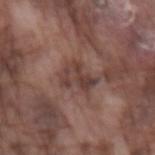follow-up=catalogued during a skin exam; not biopsied | automated lesion analysis=a classifier nevus-likeness of about 0/100 and a detector confidence of about 95 out of 100 that the crop contains a lesion | imaging modality=15 mm crop, total-body photography | lesion diameter=~4 mm (longest diameter) | patient=male, in their mid- to late 70s | tile lighting=white-light | anatomic site=the left forearm.